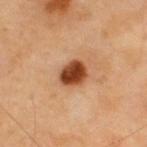{"biopsy_status": "not biopsied; imaged during a skin examination", "patient": {"sex": "male", "age_approx": 40}, "site": "upper back", "lighting": "cross-polarized", "image": {"source": "total-body photography crop", "field_of_view_mm": 15}}